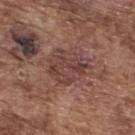notes = total-body-photography surveillance lesion; no biopsy
lesion size = ≈5 mm
image = ~15 mm crop, total-body skin-cancer survey
image-analysis metrics = an automated nevus-likeness rating near 5 out of 100 and a lesion-detection confidence of about 100/100
illumination = white-light
location = the upper back
subject = male, aged 73–77